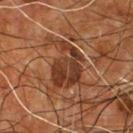follow-up: imaged on a skin check; not biopsied
anatomic site: the chest
automated lesion analysis: a lesion area of about 16 mm², an eccentricity of roughly 0.75, and two-axis asymmetry of about 0.45; border irregularity of about 7 on a 0–10 scale, a color-variation rating of about 5/10, and radial color variation of about 1.5
illumination: cross-polarized illumination
subject: male, aged approximately 55
acquisition: ~15 mm crop, total-body skin-cancer survey